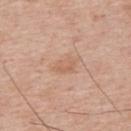Q: Is there a histopathology result?
A: catalogued during a skin exam; not biopsied
Q: Patient demographics?
A: male, aged 63 to 67
Q: Lesion location?
A: the upper back
Q: What did automated image analysis measure?
A: an area of roughly 4.5 mm²; an average lesion color of about L≈61 a*≈20 b*≈31 (CIELAB) and a lesion-to-skin contrast of about 5 (normalized; higher = more distinct); a border-irregularity index near 2.5/10, internal color variation of about 1.5 on a 0–10 scale, and peripheral color asymmetry of about 0.5; a nevus-likeness score of about 0/100 and a detector confidence of about 100 out of 100 that the crop contains a lesion
Q: How was this image acquired?
A: 15 mm crop, total-body photography
Q: How large is the lesion?
A: about 3 mm